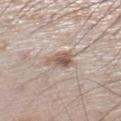workup: total-body-photography surveillance lesion; no biopsy
patient: male, aged around 60
image source: ~15 mm tile from a whole-body skin photo
body site: the left lower leg